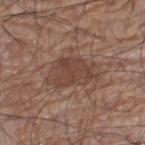A 15 mm crop from a total-body photograph taken for skin-cancer surveillance.
Measured at roughly 5.5 mm in maximum diameter.
The lesion is located on the leg.
This is a white-light tile.
A male patient, aged 68 to 72.